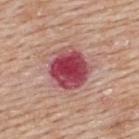Part of a total-body skin-imaging series; this lesion was reviewed on a skin check and was not flagged for biopsy. The lesion is on the upper back. A male subject aged around 60. A lesion tile, about 15 mm wide, cut from a 3D total-body photograph. Captured under white-light illumination. An algorithmic analysis of the crop reported an average lesion color of about L≈47 a*≈34 b*≈20 (CIELAB), roughly 17 lightness units darker than nearby skin, and a normalized border contrast of about 12. The software also gave a nevus-likeness score of about 0/100 and lesion-presence confidence of about 100/100.